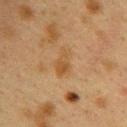Part of a total-body skin-imaging series; this lesion was reviewed on a skin check and was not flagged for biopsy. Captured under cross-polarized illumination. A lesion tile, about 15 mm wide, cut from a 3D total-body photograph. A female subject about 40 years old. Approximately 3.5 mm at its widest. Located on the upper back.